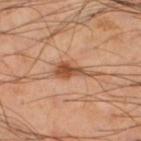| key | value |
|---|---|
| notes | imaged on a skin check; not biopsied |
| imaging modality | ~15 mm tile from a whole-body skin photo |
| patient | male, roughly 50 years of age |
| body site | the leg |